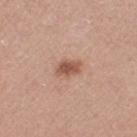No biopsy was performed on this lesion — it was imaged during a full skin examination and was not determined to be concerning. From the right thigh. A 15 mm close-up tile from a total-body photography series done for melanoma screening. The total-body-photography lesion software estimated a lesion color around L≈55 a*≈22 b*≈29 in CIELAB, a lesion–skin lightness drop of about 12, and a normalized lesion–skin contrast near 8. And it measured a classifier nevus-likeness of about 90/100 and lesion-presence confidence of about 100/100. A female subject, roughly 55 years of age. The lesion's longest dimension is about 3 mm.